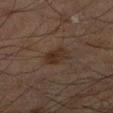{
  "biopsy_status": "not biopsied; imaged during a skin examination",
  "patient": {
    "sex": "male",
    "age_approx": 65
  },
  "site": "left lower leg",
  "image": {
    "source": "total-body photography crop",
    "field_of_view_mm": 15
  },
  "lighting": "cross-polarized",
  "lesion_size": {
    "long_diameter_mm_approx": 3.5
  }
}